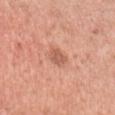The recorded lesion diameter is about 4 mm.
The total-body-photography lesion software estimated an average lesion color of about L≈62 a*≈25 b*≈31 (CIELAB), about 7 CIELAB-L* units darker than the surrounding skin, and a normalized lesion–skin contrast near 4.5. The software also gave a classifier nevus-likeness of about 55/100 and lesion-presence confidence of about 100/100.
The patient is a female in their mid- to late 30s.
The lesion is on the left upper arm.
A 15 mm close-up extracted from a 3D total-body photography capture.